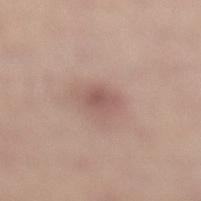Q: Was a biopsy performed?
A: total-body-photography surveillance lesion; no biopsy
Q: Patient demographics?
A: female, in their 30s
Q: What is the anatomic site?
A: the left lower leg
Q: What is the imaging modality?
A: ~15 mm tile from a whole-body skin photo
Q: What is the lesion's diameter?
A: about 2.5 mm
Q: Illumination type?
A: white-light